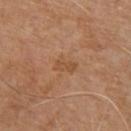Clinical impression:
No biopsy was performed on this lesion — it was imaged during a full skin examination and was not determined to be concerning.
Context:
A male patient, aged around 70. Located on the chest. A roughly 15 mm field-of-view crop from a total-body skin photograph.